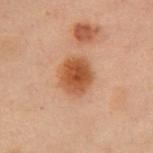About 4 mm across.
This is a cross-polarized tile.
The lesion is on the chest.
A female patient, aged approximately 55.
Automated tile analysis of the lesion measured a border-irregularity rating of about 1/10, a color-variation rating of about 4/10, and peripheral color asymmetry of about 1.
A 15 mm crop from a total-body photograph taken for skin-cancer surveillance.